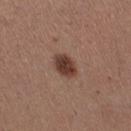follow-up = total-body-photography surveillance lesion; no biopsy
image = total-body-photography crop, ~15 mm field of view
subject = female, approximately 30 years of age
anatomic site = the right thigh